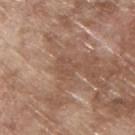Imaged during a routine full-body skin examination; the lesion was not biopsied and no histopathology is available. Approximately 3 mm at its widest. The tile uses white-light illumination. The patient is a male approximately 65 years of age. The lesion is located on the upper back. A lesion tile, about 15 mm wide, cut from a 3D total-body photograph.